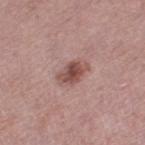The lesion was tiled from a total-body skin photograph and was not biopsied.
The subject is a female aged around 70.
Approximately 3.5 mm at its widest.
Cropped from a total-body skin-imaging series; the visible field is about 15 mm.
Located on the left thigh.